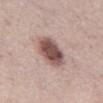No biopsy was performed on this lesion — it was imaged during a full skin examination and was not determined to be concerning.
This is a white-light tile.
A male subject approximately 65 years of age.
From the chest.
The recorded lesion diameter is about 4.5 mm.
Automated image analysis of the tile measured an outline eccentricity of about 0.55 (0 = round, 1 = elongated) and two-axis asymmetry of about 0.15. The analysis additionally found an average lesion color of about L≈52 a*≈20 b*≈21 (CIELAB), about 16 CIELAB-L* units darker than the surrounding skin, and a lesion-to-skin contrast of about 11 (normalized; higher = more distinct). The analysis additionally found border irregularity of about 1.5 on a 0–10 scale, a within-lesion color-variation index near 5/10, and radial color variation of about 1.5.
A lesion tile, about 15 mm wide, cut from a 3D total-body photograph.